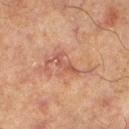follow-up — imaged on a skin check; not biopsied
TBP lesion metrics — an area of roughly 3.5 mm², an eccentricity of roughly 0.9, and a shape-asymmetry score of about 0.5 (0 = symmetric); an average lesion color of about L≈43 a*≈21 b*≈24 (CIELAB) and about 7 CIELAB-L* units darker than the surrounding skin
site — the right lower leg
subject — male, approximately 65 years of age
illumination — cross-polarized
diameter — about 3.5 mm
acquisition — 15 mm crop, total-body photography